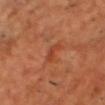workup: no biopsy performed (imaged during a skin exam) | image-analysis metrics: a footprint of about 3 mm², a shape eccentricity near 0.95, and two-axis asymmetry of about 0.5; a mean CIELAB color near L≈44 a*≈31 b*≈37 and a normalized border contrast of about 5.5; a classifier nevus-likeness of about 0/100 and a lesion-detection confidence of about 95/100 | subject: male, in their 50s | location: the front of the torso | image: ~15 mm crop, total-body skin-cancer survey | illumination: cross-polarized illumination | lesion diameter: about 3 mm.